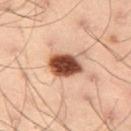Recorded during total-body skin imaging; not selected for excision or biopsy. A 15 mm close-up tile from a total-body photography series done for melanoma screening. Automated tile analysis of the lesion measured a lesion color around L≈40 a*≈20 b*≈27 in CIELAB, roughly 20 lightness units darker than nearby skin, and a lesion-to-skin contrast of about 15 (normalized; higher = more distinct). And it measured a border-irregularity index near 1.5/10, internal color variation of about 5.5 on a 0–10 scale, and radial color variation of about 1.5. And it measured an automated nevus-likeness rating near 100 out of 100. A male subject roughly 55 years of age. The lesion is located on the right thigh.